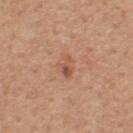<lesion>
<biopsy_status>not biopsied; imaged during a skin examination</biopsy_status>
<image>
  <source>total-body photography crop</source>
  <field_of_view_mm>15</field_of_view_mm>
</image>
<site>upper back</site>
<automated_metrics>
  <eccentricity>0.7</eccentricity>
  <shape_asymmetry>0.3</shape_asymmetry>
  <cielab_L>54</cielab_L>
  <cielab_a>23</cielab_a>
  <cielab_b>31</cielab_b>
  <vs_skin_darker_L>8.0</vs_skin_darker_L>
  <vs_skin_contrast_norm>6.0</vs_skin_contrast_norm>
  <color_variation_0_10>5.0</color_variation_0_10>
  <peripheral_color_asymmetry>2.0</peripheral_color_asymmetry>
</automated_metrics>
<lighting>white-light</lighting>
<lesion_size>
  <long_diameter_mm_approx>2.5</long_diameter_mm_approx>
</lesion_size>
<patient>
  <sex>male</sex>
  <age_approx>50</age_approx>
</patient>
</lesion>